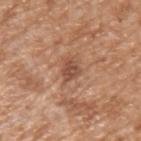follow-up — no biopsy performed (imaged during a skin exam) | size — ~2.5 mm (longest diameter) | anatomic site — the left upper arm | patient — male, in their mid-60s | illumination — white-light | acquisition — ~15 mm crop, total-body skin-cancer survey.